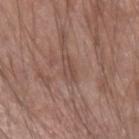This lesion was catalogued during total-body skin photography and was not selected for biopsy. A 15 mm close-up tile from a total-body photography series done for melanoma screening. A male patient, aged 53–57. The lesion is on the left forearm.